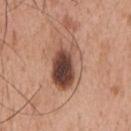  biopsy_status: not biopsied; imaged during a skin examination
  image:
    source: total-body photography crop
    field_of_view_mm: 15
  patient:
    sex: male
    age_approx: 55
  site: chest
  lighting: white-light
  lesion_size:
    long_diameter_mm_approx: 6.0